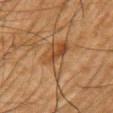{"biopsy_status": "not biopsied; imaged during a skin examination", "automated_metrics": {"area_mm2_approx": 10.0, "eccentricity": 0.9, "shape_asymmetry": 0.5}, "site": "left upper arm", "lighting": "cross-polarized", "patient": {"sex": "male", "age_approx": 65}, "lesion_size": {"long_diameter_mm_approx": 6.0}, "image": {"source": "total-body photography crop", "field_of_view_mm": 15}}